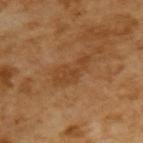Findings:
- biopsy status: imaged on a skin check; not biopsied
- image: 15 mm crop, total-body photography
- patient: male, roughly 65 years of age